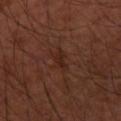Part of a total-body skin-imaging series; this lesion was reviewed on a skin check and was not flagged for biopsy. Located on the left arm. Cropped from a whole-body photographic skin survey; the tile spans about 15 mm. The total-body-photography lesion software estimated a normalized lesion–skin contrast near 6. Imaged with cross-polarized lighting. Approximately 3 mm at its widest. A male subject, about 50 years old.